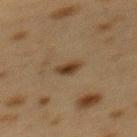Imaged during a routine full-body skin examination; the lesion was not biopsied and no histopathology is available.
About 2.5 mm across.
A close-up tile cropped from a whole-body skin photograph, about 15 mm across.
The patient is a female aged 38 to 42.
Automated tile analysis of the lesion measured an area of roughly 3.5 mm², an outline eccentricity of about 0.75 (0 = round, 1 = elongated), and a symmetry-axis asymmetry near 0.25.
The lesion is on the mid back.